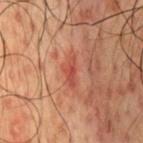Impression:
Recorded during total-body skin imaging; not selected for excision or biopsy.
Acquisition and patient details:
A 15 mm crop from a total-body photograph taken for skin-cancer surveillance. A male subject, in their 50s. This is a cross-polarized tile. Located on the chest. The lesion's longest dimension is about 3.5 mm.